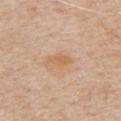workup = no biopsy performed (imaged during a skin exam)
acquisition = ~15 mm tile from a whole-body skin photo
subject = male, in their 60s
automated metrics = an area of roughly 3.5 mm², an eccentricity of roughly 0.9, and a symmetry-axis asymmetry near 0.2; border irregularity of about 2 on a 0–10 scale and a peripheral color-asymmetry measure near 0.5
anatomic site = the chest
size = ≈3 mm